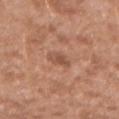patient: female, in their 40s
automated metrics: an outline eccentricity of about 0.65 (0 = round, 1 = elongated); a mean CIELAB color near L≈52 a*≈23 b*≈30, roughly 8 lightness units darker than nearby skin, and a lesion-to-skin contrast of about 6 (normalized; higher = more distinct); a border-irregularity rating of about 2/10, internal color variation of about 3 on a 0–10 scale, and a peripheral color-asymmetry measure near 1
lighting: white-light illumination
image: ~15 mm tile from a whole-body skin photo
location: the left forearm